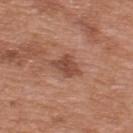Q: Was this lesion biopsied?
A: total-body-photography surveillance lesion; no biopsy
Q: What are the patient's age and sex?
A: male, aged around 70
Q: How was this image acquired?
A: ~15 mm crop, total-body skin-cancer survey
Q: Lesion size?
A: ~3.5 mm (longest diameter)
Q: Lesion location?
A: the upper back